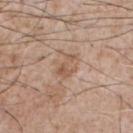Assessment: No biopsy was performed on this lesion — it was imaged during a full skin examination and was not determined to be concerning. Context: A male subject, approximately 75 years of age. The lesion is located on the chest. The lesion's longest dimension is about 3 mm. A region of skin cropped from a whole-body photographic capture, roughly 15 mm wide. The tile uses white-light illumination.